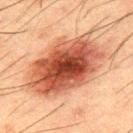No biopsy was performed on this lesion — it was imaged during a full skin examination and was not determined to be concerning.
A male patient, aged 48–52.
The tile uses cross-polarized illumination.
This image is a 15 mm lesion crop taken from a total-body photograph.
The lesion is on the mid back.